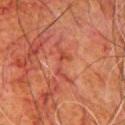Q: Patient demographics?
A: male, approximately 80 years of age
Q: Where on the body is the lesion?
A: the chest
Q: What lighting was used for the tile?
A: cross-polarized illumination
Q: What kind of image is this?
A: total-body-photography crop, ~15 mm field of view
Q: What did automated image analysis measure?
A: a lesion area of about 5 mm²; an average lesion color of about L≈38 a*≈28 b*≈27 (CIELAB), a lesion–skin lightness drop of about 6, and a lesion-to-skin contrast of about 5 (normalized; higher = more distinct)
Q: What is the lesion's diameter?
A: about 5 mm